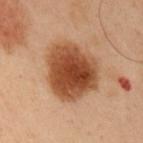Part of a total-body skin-imaging series; this lesion was reviewed on a skin check and was not flagged for biopsy.
Approximately 7 mm at its widest.
The subject is a male in their mid-50s.
The tile uses cross-polarized illumination.
Located on the right upper arm.
A roughly 15 mm field-of-view crop from a total-body skin photograph.